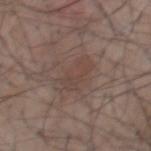The lesion was tiled from a total-body skin photograph and was not biopsied.
The lesion's longest dimension is about 4.5 mm.
A 15 mm close-up tile from a total-body photography series done for melanoma screening.
A male subject, in their mid- to late 50s.
From the front of the torso.